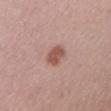Recorded during total-body skin imaging; not selected for excision or biopsy. About 3 mm across. The tile uses white-light illumination. Cropped from a whole-body photographic skin survey; the tile spans about 15 mm. The lesion is located on the right upper arm. The subject is a female aged around 45.